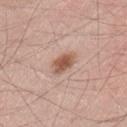Imaged during a routine full-body skin examination; the lesion was not biopsied and no histopathology is available. A lesion tile, about 15 mm wide, cut from a 3D total-body photograph. A male subject roughly 45 years of age. On the left thigh.